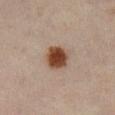<record>
  <biopsy_status>not biopsied; imaged during a skin examination</biopsy_status>
  <patient>
    <sex>female</sex>
    <age_approx>45</age_approx>
  </patient>
  <image>
    <source>total-body photography crop</source>
    <field_of_view_mm>15</field_of_view_mm>
  </image>
  <lighting>cross-polarized</lighting>
  <lesion_size>
    <long_diameter_mm_approx>3.5</long_diameter_mm_approx>
  </lesion_size>
  <site>right lower leg</site>
</record>